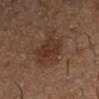Part of a total-body skin-imaging series; this lesion was reviewed on a skin check and was not flagged for biopsy.
Longest diameter approximately 5 mm.
From the left forearm.
A region of skin cropped from a whole-body photographic capture, roughly 15 mm wide.
The patient is a male approximately 55 years of age.